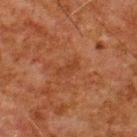Acquisition and patient details: About 3 mm across. Cropped from a total-body skin-imaging series; the visible field is about 15 mm. The lesion is located on the upper back. The tile uses cross-polarized illumination. The subject is a male aged 78–82. Automated image analysis of the tile measured a lesion color around L≈32 a*≈21 b*≈29 in CIELAB and a normalized border contrast of about 4.5. The software also gave internal color variation of about 0.5 on a 0–10 scale and radial color variation of about 0.